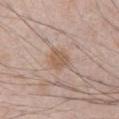Q: Was this lesion biopsied?
A: catalogued during a skin exam; not biopsied
Q: What are the patient's age and sex?
A: male, aged around 70
Q: What did automated image analysis measure?
A: a lesion area of about 5.5 mm², a shape eccentricity near 0.4, and a shape-asymmetry score of about 0.3 (0 = symmetric); a mean CIELAB color near L≈57 a*≈17 b*≈28, a lesion–skin lightness drop of about 8, and a normalized border contrast of about 6.5; internal color variation of about 3 on a 0–10 scale and peripheral color asymmetry of about 1; an automated nevus-likeness rating near 55 out of 100
Q: What is the anatomic site?
A: the chest
Q: What kind of image is this?
A: total-body-photography crop, ~15 mm field of view
Q: Lesion size?
A: ≈3 mm
Q: Illumination type?
A: white-light